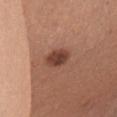| feature | finding |
|---|---|
| follow-up | total-body-photography surveillance lesion; no biopsy |
| illumination | white-light |
| diameter | ~3 mm (longest diameter) |
| imaging modality | ~15 mm crop, total-body skin-cancer survey |
| patient | female, in their mid- to late 50s |
| body site | the right upper arm |